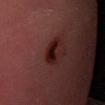Findings:
- biopsy status · no biopsy performed (imaged during a skin exam)